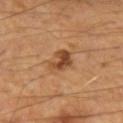Clinical impression: Captured during whole-body skin photography for melanoma surveillance; the lesion was not biopsied. Background: From the left forearm. The subject is a male about 45 years old. Cropped from a whole-body photographic skin survey; the tile spans about 15 mm. The tile uses cross-polarized illumination.